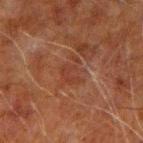Findings:
• workup: total-body-photography surveillance lesion; no biopsy
• patient: male, approximately 60 years of age
• size: about 3 mm
• site: the left arm
• illumination: cross-polarized
• image: ~15 mm tile from a whole-body skin photo
• image-analysis metrics: a footprint of about 6 mm² and a shape eccentricity near 0.65; a border-irregularity rating of about 6/10, internal color variation of about 1.5 on a 0–10 scale, and a peripheral color-asymmetry measure near 0.5; an automated nevus-likeness rating near 0 out of 100